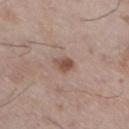Q: Is there a histopathology result?
A: catalogued during a skin exam; not biopsied
Q: What lighting was used for the tile?
A: white-light illumination
Q: What is the imaging modality?
A: total-body-photography crop, ~15 mm field of view
Q: Patient demographics?
A: male, roughly 60 years of age
Q: Where on the body is the lesion?
A: the leg